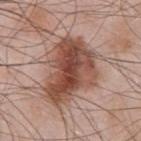No biopsy was performed on this lesion — it was imaged during a full skin examination and was not determined to be concerning. The patient is a male roughly 55 years of age. From the front of the torso. Captured under white-light illumination. This image is a 15 mm lesion crop taken from a total-body photograph.